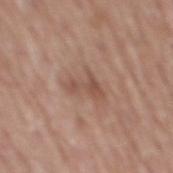Impression:
Captured during whole-body skin photography for melanoma surveillance; the lesion was not biopsied.
Background:
Approximately 3.5 mm at its widest. Cropped from a total-body skin-imaging series; the visible field is about 15 mm. A male subject, aged 73 to 77. From the back.